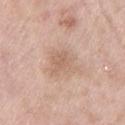Impression:
The lesion was photographed on a routine skin check and not biopsied; there is no pathology result.
Context:
The lesion is located on the leg. A female patient aged around 55. A 15 mm close-up extracted from a 3D total-body photography capture. Captured under white-light illumination. The lesion-visualizer software estimated a footprint of about 10 mm², an eccentricity of roughly 0.55, and two-axis asymmetry of about 0.2. It also reported a lesion–skin lightness drop of about 7 and a lesion-to-skin contrast of about 5 (normalized; higher = more distinct). The analysis additionally found an automated nevus-likeness rating near 0 out of 100 and a detector confidence of about 100 out of 100 that the crop contains a lesion.